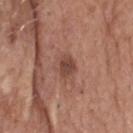Captured during whole-body skin photography for melanoma surveillance; the lesion was not biopsied. Located on the head or neck. A lesion tile, about 15 mm wide, cut from a 3D total-body photograph. The patient is a male aged 63 to 67.